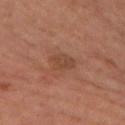Clinical impression: No biopsy was performed on this lesion — it was imaged during a full skin examination and was not determined to be concerning. Background: The lesion is located on the left lower leg. A close-up tile cropped from a whole-body skin photograph, about 15 mm across. A female subject in their mid- to late 60s.